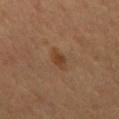The lesion was photographed on a routine skin check and not biopsied; there is no pathology result. The subject is a male in their mid-50s. The lesion is on the mid back. A lesion tile, about 15 mm wide, cut from a 3D total-body photograph. Longest diameter approximately 3.5 mm. The tile uses cross-polarized illumination.